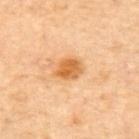workup: catalogued during a skin exam; not biopsied | anatomic site: the upper back | illumination: cross-polarized illumination | patient: male, approximately 55 years of age | image source: 15 mm crop, total-body photography | size: ~3.5 mm (longest diameter) | image-analysis metrics: a footprint of about 7 mm², a shape eccentricity near 0.65, and a shape-asymmetry score of about 0.2 (0 = symmetric); roughly 12 lightness units darker than nearby skin and a normalized border contrast of about 8.5; border irregularity of about 1.5 on a 0–10 scale, a color-variation rating of about 4.5/10, and peripheral color asymmetry of about 1.5; a nevus-likeness score of about 95/100 and a detector confidence of about 100 out of 100 that the crop contains a lesion.